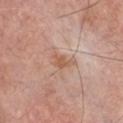workup: no biopsy performed (imaged during a skin exam) | patient: male, roughly 60 years of age | tile lighting: white-light | automated metrics: a classifier nevus-likeness of about 0/100 and a detector confidence of about 100 out of 100 that the crop contains a lesion | lesion size: about 2.5 mm | location: the chest | acquisition: total-body-photography crop, ~15 mm field of view.